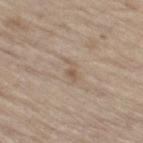| field | value |
|---|---|
| workup | no biopsy performed (imaged during a skin exam) |
| acquisition | ~15 mm tile from a whole-body skin photo |
| patient | male, in their 70s |
| site | the right thigh |